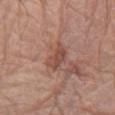Notes:
- workup · catalogued during a skin exam; not biopsied
- lesion diameter · ≈3.5 mm
- TBP lesion metrics · a footprint of about 7 mm², a shape eccentricity near 0.8, and a symmetry-axis asymmetry near 0.35
- tile lighting · white-light illumination
- subject · male, in their 80s
- imaging modality · total-body-photography crop, ~15 mm field of view
- location · the left forearm